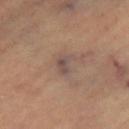{
  "biopsy_status": "not biopsied; imaged during a skin examination",
  "lesion_size": {
    "long_diameter_mm_approx": 2.5
  },
  "automated_metrics": {
    "cielab_L": 50,
    "cielab_a": 16,
    "cielab_b": 22,
    "vs_skin_darker_L": 7.0,
    "vs_skin_contrast_norm": 7.0,
    "border_irregularity_0_10": 3.5,
    "peripheral_color_asymmetry": 1.0,
    "nevus_likeness_0_100": 0,
    "lesion_detection_confidence_0_100": 90
  },
  "patient": {
    "sex": "female",
    "age_approx": 70
  },
  "site": "right thigh",
  "lighting": "cross-polarized",
  "image": {
    "source": "total-body photography crop",
    "field_of_view_mm": 15
  }
}